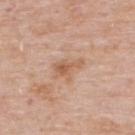The lesion is located on the upper back.
A male patient about 75 years old.
Measured at roughly 3.5 mm in maximum diameter.
Automated image analysis of the tile measured lesion-presence confidence of about 100/100.
This is a white-light tile.
Cropped from a whole-body photographic skin survey; the tile spans about 15 mm.